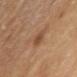The lesion was photographed on a routine skin check and not biopsied; there is no pathology result. The recorded lesion diameter is about 3.5 mm. The patient is a female about 55 years old. Located on the left upper arm. A 15 mm close-up extracted from a 3D total-body photography capture.